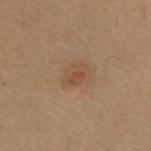workup: no biopsy performed (imaged during a skin exam) | image-analysis metrics: an area of roughly 5.5 mm² and a symmetry-axis asymmetry near 0.2; about 6 CIELAB-L* units darker than the surrounding skin and a lesion-to-skin contrast of about 5.5 (normalized; higher = more distinct); a border-irregularity rating of about 2/10, a color-variation rating of about 2.5/10, and radial color variation of about 1; an automated nevus-likeness rating near 60 out of 100 and a detector confidence of about 100 out of 100 that the crop contains a lesion | size: ≈3 mm | acquisition: 15 mm crop, total-body photography | lighting: cross-polarized illumination | subject: female, aged 48–52 | body site: the chest.